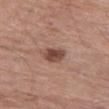Part of a total-body skin-imaging series; this lesion was reviewed on a skin check and was not flagged for biopsy.
A 15 mm close-up tile from a total-body photography series done for melanoma screening.
Longest diameter approximately 3 mm.
On the left thigh.
The patient is a male aged 78–82.
An algorithmic analysis of the crop reported an outline eccentricity of about 0.45 (0 = round, 1 = elongated) and a symmetry-axis asymmetry near 0.2. The software also gave a border-irregularity index near 2/10 and peripheral color asymmetry of about 1.5.